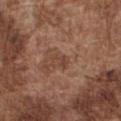Findings:
• follow-up · catalogued during a skin exam; not biopsied
• imaging modality · total-body-photography crop, ~15 mm field of view
• body site · the front of the torso
• illumination · white-light illumination
• patient · male, aged approximately 75
• lesion diameter · ~2.5 mm (longest diameter)